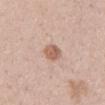Impression:
No biopsy was performed on this lesion — it was imaged during a full skin examination and was not determined to be concerning.
Background:
Imaged with white-light lighting. Automated tile analysis of the lesion measured a footprint of about 5 mm² and an eccentricity of roughly 0.65. The analysis additionally found a lesion color around L≈60 a*≈20 b*≈28 in CIELAB, a lesion–skin lightness drop of about 12, and a lesion-to-skin contrast of about 7.5 (normalized; higher = more distinct). The analysis additionally found a border-irregularity index near 1.5/10, a color-variation rating of about 2.5/10, and radial color variation of about 1. And it measured a nevus-likeness score of about 70/100 and a detector confidence of about 100 out of 100 that the crop contains a lesion. The lesion is located on the mid back. About 3 mm across. The patient is a male approximately 40 years of age. A 15 mm close-up tile from a total-body photography series done for melanoma screening.